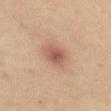This lesion was catalogued during total-body skin photography and was not selected for biopsy. Longest diameter approximately 3 mm. Located on the left thigh. A female subject, in their 40s. Imaged with cross-polarized lighting. A 15 mm close-up extracted from a 3D total-body photography capture. The total-body-photography lesion software estimated an area of roughly 6 mm² and an outline eccentricity of about 0.65 (0 = round, 1 = elongated). And it measured a mean CIELAB color near L≈44 a*≈19 b*≈22, about 9 CIELAB-L* units darker than the surrounding skin, and a lesion-to-skin contrast of about 7.5 (normalized; higher = more distinct). The analysis additionally found a classifier nevus-likeness of about 80/100 and lesion-presence confidence of about 100/100.